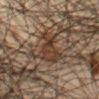Impression: The lesion was photographed on a routine skin check and not biopsied; there is no pathology result. Acquisition and patient details: A male patient, aged 43 to 47. Longest diameter approximately 4 mm. On the front of the torso. A 15 mm close-up extracted from a 3D total-body photography capture. Imaged with cross-polarized lighting.